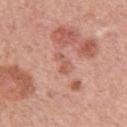No biopsy was performed on this lesion — it was imaged during a full skin examination and was not determined to be concerning.